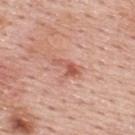workup: no biopsy performed (imaged during a skin exam)
tile lighting: white-light illumination
diameter: ≈3.5 mm
patient: male, aged 53 to 57
automated lesion analysis: an outline eccentricity of about 0.85 (0 = round, 1 = elongated); a lesion color around L≈58 a*≈27 b*≈30 in CIELAB; a classifier nevus-likeness of about 25/100
site: the upper back
image source: total-body-photography crop, ~15 mm field of view